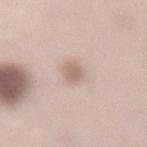Impression: The lesion was photographed on a routine skin check and not biopsied; there is no pathology result. Image and clinical context: The lesion's longest dimension is about 3 mm. Cropped from a whole-body photographic skin survey; the tile spans about 15 mm. On the lower back. The patient is a female roughly 40 years of age. The tile uses white-light illumination. Automated tile analysis of the lesion measured a shape eccentricity near 0.9 and two-axis asymmetry of about 0.25. It also reported a within-lesion color-variation index near 2/10 and peripheral color asymmetry of about 0.5. The analysis additionally found a nevus-likeness score of about 100/100 and a lesion-detection confidence of about 100/100.